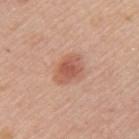Clinical impression:
No biopsy was performed on this lesion — it was imaged during a full skin examination and was not determined to be concerning.